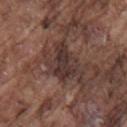The lesion was tiled from a total-body skin photograph and was not biopsied.
The subject is a male about 75 years old.
A 15 mm crop from a total-body photograph taken for skin-cancer surveillance.
The lesion is on the left upper arm.
Longest diameter approximately 4.5 mm.
This is a white-light tile.